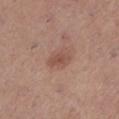Impression: Captured during whole-body skin photography for melanoma surveillance; the lesion was not biopsied. Image and clinical context: A female subject, aged 43 to 47. Longest diameter approximately 3 mm. Captured under white-light illumination. Located on the left lower leg. A roughly 15 mm field-of-view crop from a total-body skin photograph.